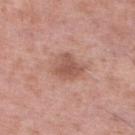Assessment:
This lesion was catalogued during total-body skin photography and was not selected for biopsy.
Acquisition and patient details:
A close-up tile cropped from a whole-body skin photograph, about 15 mm across. The lesion is located on the right lower leg. Captured under white-light illumination. About 3.5 mm across. The total-body-photography lesion software estimated a lesion color around L≈55 a*≈23 b*≈27 in CIELAB and a normalized border contrast of about 7. The software also gave a border-irregularity index near 2.5/10. And it measured a nevus-likeness score of about 60/100. A male subject, aged 53–57.